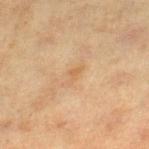Clinical impression: The lesion was tiled from a total-body skin photograph and was not biopsied. Context: The total-body-photography lesion software estimated an eccentricity of roughly 0.85 and a symmetry-axis asymmetry near 0.45. It also reported a border-irregularity index near 5/10 and radial color variation of about 0. Imaged with cross-polarized lighting. A close-up tile cropped from a whole-body skin photograph, about 15 mm across. From the right lower leg. The subject is a female aged approximately 40. The recorded lesion diameter is about 2.5 mm.